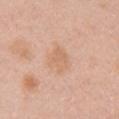Q: Was a biopsy performed?
A: catalogued during a skin exam; not biopsied
Q: What is the anatomic site?
A: the right upper arm
Q: How large is the lesion?
A: about 2.5 mm
Q: Automated lesion metrics?
A: an eccentricity of roughly 0.7
Q: Illumination type?
A: white-light
Q: Patient demographics?
A: female, aged around 50
Q: How was this image acquired?
A: 15 mm crop, total-body photography